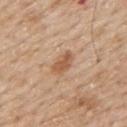Impression: No biopsy was performed on this lesion — it was imaged during a full skin examination and was not determined to be concerning. Image and clinical context: The lesion's longest dimension is about 3.5 mm. A close-up tile cropped from a whole-body skin photograph, about 15 mm across. Captured under white-light illumination. A male patient roughly 60 years of age. Located on the upper back.